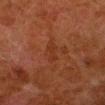Part of a total-body skin-imaging series; this lesion was reviewed on a skin check and was not flagged for biopsy.
A 15 mm close-up tile from a total-body photography series done for melanoma screening.
Located on the leg.
The subject is a male aged around 80.
The tile uses cross-polarized illumination.